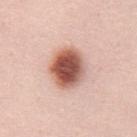Recorded during total-body skin imaging; not selected for excision or biopsy.
Cropped from a total-body skin-imaging series; the visible field is about 15 mm.
A male subject roughly 35 years of age.
The lesion is located on the abdomen.
About 5 mm across.